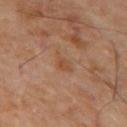Imaged during a routine full-body skin examination; the lesion was not biopsied and no histopathology is available. The lesion is on the mid back. Longest diameter approximately 2.5 mm. Automated image analysis of the tile measured a footprint of about 3 mm² and a symmetry-axis asymmetry near 0.35. And it measured an average lesion color of about L≈44 a*≈20 b*≈30 (CIELAB), roughly 6 lightness units darker than nearby skin, and a lesion-to-skin contrast of about 5.5 (normalized; higher = more distinct). The software also gave a border-irregularity index near 3.5/10, a color-variation rating of about 2/10, and peripheral color asymmetry of about 0.5. The tile uses cross-polarized illumination. A male subject, about 60 years old. A 15 mm close-up tile from a total-body photography series done for melanoma screening.